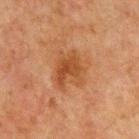Impression: This lesion was catalogued during total-body skin photography and was not selected for biopsy. Context: The lesion's longest dimension is about 5 mm. A 15 mm crop from a total-body photograph taken for skin-cancer surveillance. A male patient aged approximately 65. An algorithmic analysis of the crop reported a mean CIELAB color near L≈40 a*≈21 b*≈32 and a lesion–skin lightness drop of about 7. The analysis additionally found radial color variation of about 1.5. Imaged with cross-polarized lighting. From the front of the torso.